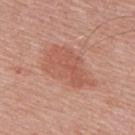This lesion was catalogued during total-body skin photography and was not selected for biopsy. About 6.5 mm across. This is a white-light tile. A region of skin cropped from a whole-body photographic capture, roughly 15 mm wide. The total-body-photography lesion software estimated an area of roughly 18 mm² and a shape-asymmetry score of about 0.3 (0 = symmetric). The analysis additionally found a lesion color around L≈56 a*≈25 b*≈30 in CIELAB and a lesion-to-skin contrast of about 5.5 (normalized; higher = more distinct). The analysis additionally found border irregularity of about 3.5 on a 0–10 scale, internal color variation of about 3 on a 0–10 scale, and radial color variation of about 1. A male subject aged around 50. On the upper back.